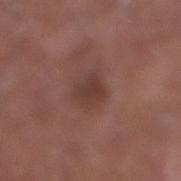notes = catalogued during a skin exam; not biopsied
lighting = white-light
anatomic site = the right lower leg
subject = male, aged approximately 55
image = total-body-photography crop, ~15 mm field of view
diameter = ≈3 mm